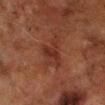No biopsy was performed on this lesion — it was imaged during a full skin examination and was not determined to be concerning. A 15 mm close-up tile from a total-body photography series done for melanoma screening. A male patient aged 68 to 72. Located on the right lower leg.